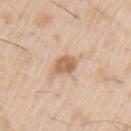body site=the arm | acquisition=15 mm crop, total-body photography | patient=male, approximately 50 years of age.